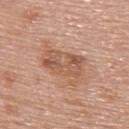{"biopsy_status": "not biopsied; imaged during a skin examination", "image": {"source": "total-body photography crop", "field_of_view_mm": 15}, "patient": {"sex": "female", "age_approx": 60}, "site": "back", "lighting": "white-light", "lesion_size": {"long_diameter_mm_approx": 5.5}}